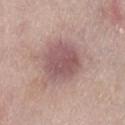Findings:
• workup — total-body-photography surveillance lesion; no biopsy
• size — ~5 mm (longest diameter)
• lighting — white-light illumination
• acquisition — ~15 mm crop, total-body skin-cancer survey
• subject — female, in their mid-60s
• anatomic site — the right lower leg
• TBP lesion metrics — about 11 CIELAB-L* units darker than the surrounding skin and a normalized border contrast of about 8; a border-irregularity index near 1.5/10 and a within-lesion color-variation index near 3.5/10; a detector confidence of about 100 out of 100 that the crop contains a lesion